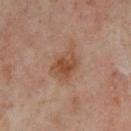Captured during whole-body skin photography for melanoma surveillance; the lesion was not biopsied. Located on the leg. A female patient, approximately 50 years of age. The lesion's longest dimension is about 3.5 mm. Cropped from a whole-body photographic skin survey; the tile spans about 15 mm.